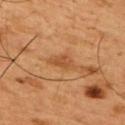<tbp_lesion>
  <biopsy_status>not biopsied; imaged during a skin examination</biopsy_status>
  <site>upper back</site>
  <lighting>cross-polarized</lighting>
  <lesion_size>
    <long_diameter_mm_approx>3.0</long_diameter_mm_approx>
  </lesion_size>
  <patient>
    <sex>male</sex>
    <age_approx>50</age_approx>
  </patient>
  <image>
    <source>total-body photography crop</source>
    <field_of_view_mm>15</field_of_view_mm>
  </image>
</tbp_lesion>